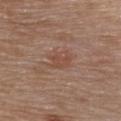notes: total-body-photography surveillance lesion; no biopsy | location: the chest | automated lesion analysis: internal color variation of about 2 on a 0–10 scale and radial color variation of about 0.5; lesion-presence confidence of about 100/100 | patient: male, in their 80s | size: ~3.5 mm (longest diameter) | imaging modality: ~15 mm crop, total-body skin-cancer survey.